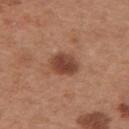| feature | finding |
|---|---|
| follow-up | no biopsy performed (imaged during a skin exam) |
| subject | male, aged around 30 |
| body site | the upper back |
| image source | 15 mm crop, total-body photography |
| size | ≈3.5 mm |
| tile lighting | white-light illumination |